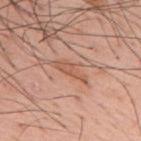Assessment:
Imaged during a routine full-body skin examination; the lesion was not biopsied and no histopathology is available.
Context:
The recorded lesion diameter is about 4 mm. A male patient, roughly 55 years of age. A 15 mm close-up extracted from a 3D total-body photography capture. Located on the upper back. Automated tile analysis of the lesion measured internal color variation of about 1 on a 0–10 scale and radial color variation of about 0.5. The analysis additionally found a classifier nevus-likeness of about 10/100 and lesion-presence confidence of about 95/100.